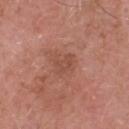lesion diameter — about 2.5 mm | illumination — white-light | automated metrics — an outline eccentricity of about 0.6 (0 = round, 1 = elongated) and a shape-asymmetry score of about 0.25 (0 = symmetric); a lesion color around L≈49 a*≈25 b*≈29 in CIELAB, about 6 CIELAB-L* units darker than the surrounding skin, and a normalized lesion–skin contrast near 4.5 | image source — ~15 mm crop, total-body skin-cancer survey | location — the head or neck | patient — male, in their mid-50s.